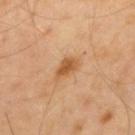biopsy status: total-body-photography surveillance lesion; no biopsy | TBP lesion metrics: an eccentricity of roughly 0.8 and a symmetry-axis asymmetry near 0.3; about 11 CIELAB-L* units darker than the surrounding skin; a border-irregularity rating of about 3/10, internal color variation of about 2.5 on a 0–10 scale, and a peripheral color-asymmetry measure near 1; lesion-presence confidence of about 100/100 | site: the upper back | tile lighting: cross-polarized | patient: male, aged around 40 | image source: 15 mm crop, total-body photography | diameter: about 3 mm.